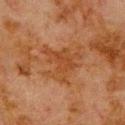| key | value |
|---|---|
| patient | male, approximately 80 years of age |
| imaging modality | ~15 mm crop, total-body skin-cancer survey |
| lighting | cross-polarized illumination |
| lesion diameter | ≈5.5 mm |
| anatomic site | the upper back |